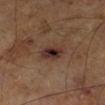Captured during whole-body skin photography for melanoma surveillance; the lesion was not biopsied. Located on the left leg. About 3 mm across. A close-up tile cropped from a whole-body skin photograph, about 15 mm across. A male subject, in their mid-60s. Imaged with cross-polarized lighting.